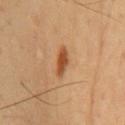{
  "biopsy_status": "not biopsied; imaged during a skin examination",
  "lesion_size": {
    "long_diameter_mm_approx": 4.0
  },
  "automated_metrics": {
    "area_mm2_approx": 5.0,
    "eccentricity": 0.9,
    "cielab_L": 51,
    "cielab_a": 24,
    "cielab_b": 39,
    "vs_skin_darker_L": 12.0,
    "vs_skin_contrast_norm": 9.0,
    "border_irregularity_0_10": 2.5,
    "color_variation_0_10": 2.0,
    "peripheral_color_asymmetry": 0.5,
    "nevus_likeness_0_100": 100,
    "lesion_detection_confidence_0_100": 100
  },
  "patient": {
    "sex": "male",
    "age_approx": 65
  },
  "site": "chest",
  "image": {
    "source": "total-body photography crop",
    "field_of_view_mm": 15
  }
}